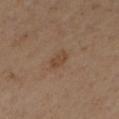Case summary:
- follow-up — total-body-photography surveillance lesion; no biopsy
- subject — male, aged 63 to 67
- lesion diameter — about 2.5 mm
- image source — total-body-photography crop, ~15 mm field of view
- tile lighting — cross-polarized illumination
- location — the left lower leg